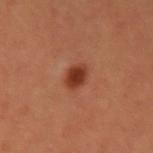acquisition: ~15 mm tile from a whole-body skin photo | patient: male, aged approximately 65 | size: about 3 mm | image-analysis metrics: a lesion area of about 5 mm², an outline eccentricity of about 0.6 (0 = round, 1 = elongated), and a symmetry-axis asymmetry near 0.15; an average lesion color of about L≈36 a*≈27 b*≈31 (CIELAB) and a lesion–skin lightness drop of about 12; a border-irregularity index near 1.5/10, a within-lesion color-variation index near 3.5/10, and a peripheral color-asymmetry measure near 1; a lesion-detection confidence of about 100/100 | tile lighting: cross-polarized illumination | body site: the left upper arm.